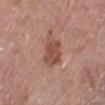Findings:
• follow-up: no biopsy performed (imaged during a skin exam)
• patient: female, in their mid-70s
• automated lesion analysis: a lesion area of about 9 mm², a shape eccentricity near 0.7, and a shape-asymmetry score of about 0.2 (0 = symmetric); border irregularity of about 2 on a 0–10 scale, internal color variation of about 2.5 on a 0–10 scale, and peripheral color asymmetry of about 1; a classifier nevus-likeness of about 20/100
• site: the left lower leg
• illumination: white-light illumination
• acquisition: ~15 mm crop, total-body skin-cancer survey